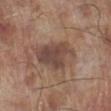Part of a total-body skin-imaging series; this lesion was reviewed on a skin check and was not flagged for biopsy. A region of skin cropped from a whole-body photographic capture, roughly 15 mm wide. The lesion's longest dimension is about 6 mm. The subject is a male roughly 70 years of age. The tile uses white-light illumination. The lesion is on the left lower leg. The total-body-photography lesion software estimated a mean CIELAB color near L≈45 a*≈17 b*≈24, roughly 10 lightness units darker than nearby skin, and a normalized lesion–skin contrast near 8. And it measured border irregularity of about 3 on a 0–10 scale and a color-variation rating of about 5/10. The software also gave a detector confidence of about 100 out of 100 that the crop contains a lesion.